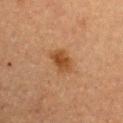workup: catalogued during a skin exam; not biopsied | acquisition: 15 mm crop, total-body photography | subject: female, aged approximately 60 | body site: the left upper arm | image-analysis metrics: an area of roughly 5.5 mm², an eccentricity of roughly 0.55, and a symmetry-axis asymmetry near 0.2; a mean CIELAB color near L≈38 a*≈20 b*≈32, about 9 CIELAB-L* units darker than the surrounding skin, and a normalized border contrast of about 8.5; a classifier nevus-likeness of about 90/100 and a lesion-detection confidence of about 100/100 | lesion size: ≈3 mm | tile lighting: cross-polarized.